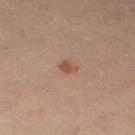subject: female, in their mid-50s
image-analysis metrics: an area of roughly 2.5 mm², an outline eccentricity of about 0.75 (0 = round, 1 = elongated), and a symmetry-axis asymmetry near 0.3; border irregularity of about 2.5 on a 0–10 scale, internal color variation of about 1 on a 0–10 scale, and a peripheral color-asymmetry measure near 0.5; a nevus-likeness score of about 85/100 and lesion-presence confidence of about 100/100
image: ~15 mm crop, total-body skin-cancer survey
site: the left thigh